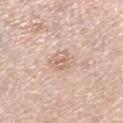{
  "biopsy_status": "not biopsied; imaged during a skin examination",
  "site": "right lower leg",
  "lighting": "white-light",
  "automated_metrics": {
    "area_mm2_approx": 6.0,
    "shape_asymmetry": 0.2,
    "cielab_L": 66,
    "cielab_a": 18,
    "cielab_b": 27,
    "vs_skin_darker_L": 8.0,
    "vs_skin_contrast_norm": 5.5,
    "nevus_likeness_0_100": 0,
    "lesion_detection_confidence_0_100": 100
  },
  "image": {
    "source": "total-body photography crop",
    "field_of_view_mm": 15
  },
  "patient": {
    "sex": "female",
    "age_approx": 65
  },
  "lesion_size": {
    "long_diameter_mm_approx": 3.5
  }
}